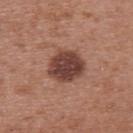{
  "biopsy_status": "not biopsied; imaged during a skin examination",
  "site": "back",
  "image": {
    "source": "total-body photography crop",
    "field_of_view_mm": 15
  },
  "lesion_size": {
    "long_diameter_mm_approx": 4.5
  },
  "automated_metrics": {
    "nevus_likeness_0_100": 45,
    "lesion_detection_confidence_0_100": 100
  },
  "patient": {
    "sex": "female",
    "age_approx": 40
  }
}